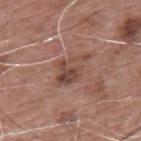No biopsy was performed on this lesion — it was imaged during a full skin examination and was not determined to be concerning.
The tile uses white-light illumination.
Automated image analysis of the tile measured a footprint of about 8 mm², a shape eccentricity near 0.85, and two-axis asymmetry of about 0.35. The analysis additionally found an average lesion color of about L≈46 a*≈20 b*≈25 (CIELAB) and a normalized border contrast of about 7. It also reported border irregularity of about 6.5 on a 0–10 scale, a within-lesion color-variation index near 5/10, and radial color variation of about 1.5. The analysis additionally found a nevus-likeness score of about 0/100 and a lesion-detection confidence of about 100/100.
This image is a 15 mm lesion crop taken from a total-body photograph.
Longest diameter approximately 4.5 mm.
Located on the upper back.
A male subject, approximately 60 years of age.